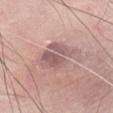No biopsy was performed on this lesion — it was imaged during a full skin examination and was not determined to be concerning. This image is a 15 mm lesion crop taken from a total-body photograph. Captured under white-light illumination. A male subject, aged 83 or over. About 4.5 mm across. The lesion is on the abdomen.